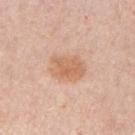This lesion was catalogued during total-body skin photography and was not selected for biopsy. Approximately 4.5 mm at its widest. The subject is a male aged approximately 60. Cropped from a whole-body photographic skin survey; the tile spans about 15 mm. On the left upper arm.